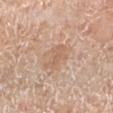Captured during whole-body skin photography for melanoma surveillance; the lesion was not biopsied.
About 3.5 mm across.
The lesion is on the left lower leg.
This is a white-light tile.
A female patient in their 70s.
This image is a 15 mm lesion crop taken from a total-body photograph.